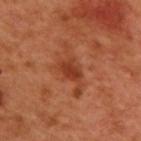Recorded during total-body skin imaging; not selected for excision or biopsy. Longest diameter approximately 2.5 mm. The lesion is located on the upper back. This is a cross-polarized tile. A male subject aged around 50. A 15 mm crop from a total-body photograph taken for skin-cancer surveillance.